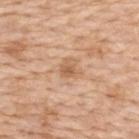Clinical impression: The lesion was tiled from a total-body skin photograph and was not biopsied. Context: A 15 mm crop from a total-body photograph taken for skin-cancer surveillance. Located on the upper back. A female patient in their mid-40s. The lesion's longest dimension is about 2.5 mm. Imaged with white-light lighting. The lesion-visualizer software estimated border irregularity of about 3.5 on a 0–10 scale and a within-lesion color-variation index near 4.5/10.